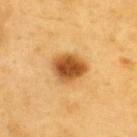Assessment:
Captured during whole-body skin photography for melanoma surveillance; the lesion was not biopsied.
Image and clinical context:
The lesion-visualizer software estimated about 18 CIELAB-L* units darker than the surrounding skin. The software also gave a border-irregularity rating of about 1.5/10 and internal color variation of about 5.5 on a 0–10 scale. And it measured an automated nevus-likeness rating near 100 out of 100 and a lesion-detection confidence of about 100/100. On the upper back. Measured at roughly 4 mm in maximum diameter. The subject is a male aged 58–62. Cropped from a total-body skin-imaging series; the visible field is about 15 mm. The tile uses cross-polarized illumination.